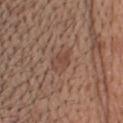| field | value |
|---|---|
| lighting | white-light illumination |
| subject | male, aged around 40 |
| anatomic site | the head or neck |
| acquisition | ~15 mm crop, total-body skin-cancer survey |